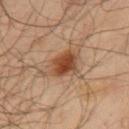| key | value |
|---|---|
| biopsy status | imaged on a skin check; not biopsied |
| illumination | cross-polarized illumination |
| acquisition | ~15 mm crop, total-body skin-cancer survey |
| subject | male, aged approximately 65 |
| location | the left upper arm |